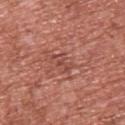biopsy_status: not biopsied; imaged during a skin examination
image:
  source: total-body photography crop
  field_of_view_mm: 15
site: chest
patient:
  sex: male
  age_approx: 45